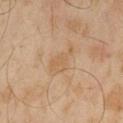Assessment:
Captured during whole-body skin photography for melanoma surveillance; the lesion was not biopsied.
Context:
The patient is a male approximately 60 years of age. A 15 mm close-up extracted from a 3D total-body photography capture. On the left thigh.